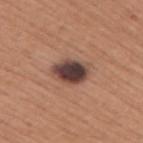subject: male, roughly 45 years of age
image: ~15 mm tile from a whole-body skin photo
site: the arm
lighting: white-light illumination
diameter: ≈4.5 mm
TBP lesion metrics: a lesion area of about 10 mm², a shape eccentricity near 0.75, and a shape-asymmetry score of about 0.2 (0 = symmetric); an average lesion color of about L≈42 a*≈18 b*≈22 (CIELAB), a lesion–skin lightness drop of about 17, and a normalized border contrast of about 14; an automated nevus-likeness rating near 60 out of 100 and lesion-presence confidence of about 100/100